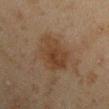Assessment: Part of a total-body skin-imaging series; this lesion was reviewed on a skin check and was not flagged for biopsy. Image and clinical context: A lesion tile, about 15 mm wide, cut from a 3D total-body photograph. A male subject aged 43 to 47. Imaged with cross-polarized lighting. An algorithmic analysis of the crop reported a lesion color around L≈32 a*≈14 b*≈25 in CIELAB, roughly 6 lightness units darker than nearby skin, and a normalized border contrast of about 7. It also reported a border-irregularity index near 2.5/10, internal color variation of about 3.5 on a 0–10 scale, and peripheral color asymmetry of about 1. It also reported a detector confidence of about 100 out of 100 that the crop contains a lesion. From the right upper arm.